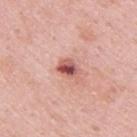This lesion was catalogued during total-body skin photography and was not selected for biopsy.
A male patient aged 48 to 52.
Measured at roughly 2.5 mm in maximum diameter.
On the upper back.
A 15 mm close-up extracted from a 3D total-body photography capture.